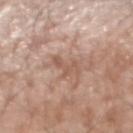Clinical impression:
Captured during whole-body skin photography for melanoma surveillance; the lesion was not biopsied.
Clinical summary:
The patient is a male aged approximately 60. Captured under white-light illumination. An algorithmic analysis of the crop reported border irregularity of about 8 on a 0–10 scale, a within-lesion color-variation index near 0/10, and radial color variation of about 0. The lesion's longest dimension is about 3.5 mm. A lesion tile, about 15 mm wide, cut from a 3D total-body photograph. The lesion is on the left forearm.